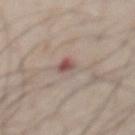notes: catalogued during a skin exam; not biopsied | body site: the chest | lighting: white-light illumination | patient: male, in their mid- to late 50s | image: ~15 mm crop, total-body skin-cancer survey | automated metrics: an average lesion color of about L≈56 a*≈15 b*≈21 (CIELAB), about 8 CIELAB-L* units darker than the surrounding skin, and a lesion-to-skin contrast of about 6 (normalized; higher = more distinct); a border-irregularity index near 4.5/10, a within-lesion color-variation index near 10/10, and peripheral color asymmetry of about 4.5.